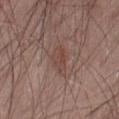The lesion was tiled from a total-body skin photograph and was not biopsied.
The lesion-visualizer software estimated an average lesion color of about L≈44 a*≈19 b*≈23 (CIELAB), roughly 6 lightness units darker than nearby skin, and a lesion-to-skin contrast of about 5.5 (normalized; higher = more distinct). It also reported a border-irregularity index near 3/10 and a color-variation rating of about 2.5/10. And it measured a classifier nevus-likeness of about 0/100.
Cropped from a total-body skin-imaging series; the visible field is about 15 mm.
Longest diameter approximately 3.5 mm.
Located on the right thigh.
The subject is a male aged 53 to 57.
The tile uses white-light illumination.